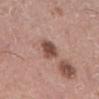workup: imaged on a skin check; not biopsied | image source: ~15 mm tile from a whole-body skin photo | tile lighting: white-light | location: the left lower leg | image-analysis metrics: about 14 CIELAB-L* units darker than the surrounding skin and a normalized border contrast of about 9.5; border irregularity of about 2 on a 0–10 scale and internal color variation of about 3.5 on a 0–10 scale | patient: male, in their 50s | size: ~3 mm (longest diameter).